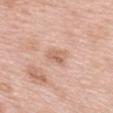<tbp_lesion>
<biopsy_status>not biopsied; imaged during a skin examination</biopsy_status>
<lighting>white-light</lighting>
<patient>
  <sex>female</sex>
  <age_approx>65</age_approx>
</patient>
<site>upper back</site>
<lesion_size>
  <long_diameter_mm_approx>2.5</long_diameter_mm_approx>
</lesion_size>
<image>
  <source>total-body photography crop</source>
  <field_of_view_mm>15</field_of_view_mm>
</image>
</tbp_lesion>